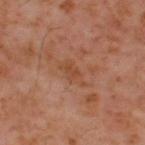• notes · no biopsy performed (imaged during a skin exam)
• subject · male, aged 58 to 62
• illumination · cross-polarized illumination
• lesion diameter · ~3.5 mm (longest diameter)
• image · ~15 mm crop, total-body skin-cancer survey
• body site · the upper back